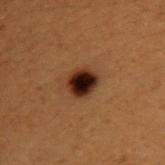| field | value |
|---|---|
| follow-up | imaged on a skin check; not biopsied |
| diameter | about 3 mm |
| patient | male, roughly 50 years of age |
| lighting | cross-polarized |
| image | total-body-photography crop, ~15 mm field of view |
| location | the upper back |
| image-analysis metrics | a mean CIELAB color near L≈19 a*≈17 b*≈20 and a normalized border contrast of about 19; border irregularity of about 1.5 on a 0–10 scale and a within-lesion color-variation index near 7.5/10; a lesion-detection confidence of about 100/100 |